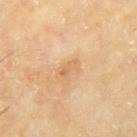| key | value |
|---|---|
| workup | imaged on a skin check; not biopsied |
| automated metrics | an area of roughly 3.5 mm² and an outline eccentricity of about 0.85 (0 = round, 1 = elongated); an average lesion color of about L≈67 a*≈19 b*≈41 (CIELAB); border irregularity of about 4 on a 0–10 scale, a color-variation rating of about 3/10, and peripheral color asymmetry of about 1 |
| subject | male, aged around 65 |
| body site | the left upper arm |
| lighting | cross-polarized illumination |
| image source | ~15 mm tile from a whole-body skin photo |